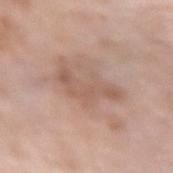Q: Is there a histopathology result?
A: no biopsy performed (imaged during a skin exam)
Q: Who is the patient?
A: female, aged approximately 75
Q: What lighting was used for the tile?
A: white-light
Q: What is the anatomic site?
A: the left forearm
Q: How was this image acquired?
A: ~15 mm tile from a whole-body skin photo